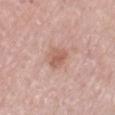workup: imaged on a skin check; not biopsied | patient: male, approximately 80 years of age | automated metrics: a lesion area of about 5 mm², an eccentricity of roughly 0.5, and a symmetry-axis asymmetry near 0.25; a mean CIELAB color near L≈59 a*≈22 b*≈27, roughly 9 lightness units darker than nearby skin, and a lesion-to-skin contrast of about 6.5 (normalized; higher = more distinct); a color-variation rating of about 2/10 and peripheral color asymmetry of about 0.5; a nevus-likeness score of about 15/100 | body site: the right thigh | diameter: ≈2.5 mm | lighting: white-light | image source: ~15 mm crop, total-body skin-cancer survey.